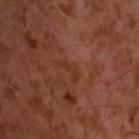The lesion was tiled from a total-body skin photograph and was not biopsied.
Captured under cross-polarized illumination.
A male patient about 60 years old.
The lesion is located on the head or neck.
A 15 mm close-up extracted from a 3D total-body photography capture.
The lesion-visualizer software estimated border irregularity of about 7 on a 0–10 scale and radial color variation of about 0. And it measured a lesion-detection confidence of about 85/100.
Measured at roughly 3.5 mm in maximum diameter.